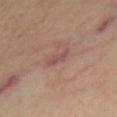Impression:
Recorded during total-body skin imaging; not selected for excision or biopsy.
Acquisition and patient details:
A 15 mm close-up extracted from a 3D total-body photography capture. A female patient approximately 50 years of age. Approximately 2.5 mm at its widest. From the right thigh. An algorithmic analysis of the crop reported an area of roughly 3 mm² and a symmetry-axis asymmetry near 0.4. And it measured an average lesion color of about L≈50 a*≈24 b*≈22 (CIELAB) and a lesion-to-skin contrast of about 6 (normalized; higher = more distinct). The analysis additionally found border irregularity of about 4 on a 0–10 scale, a color-variation rating of about 0/10, and a peripheral color-asymmetry measure near 0. The analysis additionally found a nevus-likeness score of about 0/100 and a lesion-detection confidence of about 100/100.